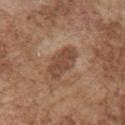Part of a total-body skin-imaging series; this lesion was reviewed on a skin check and was not flagged for biopsy.
Imaged with white-light lighting.
The lesion is located on the chest.
A 15 mm close-up extracted from a 3D total-body photography capture.
A male subject, roughly 75 years of age.
Longest diameter approximately 5 mm.